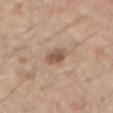site: the front of the torso
imaging modality: ~15 mm tile from a whole-body skin photo
subject: male, about 70 years old